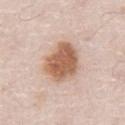notes — imaged on a skin check; not biopsied
anatomic site — the abdomen
image — ~15 mm crop, total-body skin-cancer survey
subject — male, aged around 80
lighting — white-light illumination
diameter — ≈5 mm